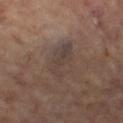This lesion was catalogued during total-body skin photography and was not selected for biopsy. Located on the right leg. Measured at roughly 4.5 mm in maximum diameter. A roughly 15 mm field-of-view crop from a total-body skin photograph. An algorithmic analysis of the crop reported an area of roughly 10 mm² and a shape eccentricity near 0.75. A female subject roughly 65 years of age.